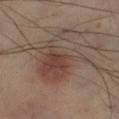No biopsy was performed on this lesion — it was imaged during a full skin examination and was not determined to be concerning.
A male patient roughly 50 years of age.
Cropped from a whole-body photographic skin survey; the tile spans about 15 mm.
Measured at roughly 8 mm in maximum diameter.
Imaged with cross-polarized lighting.
The lesion is on the right lower leg.